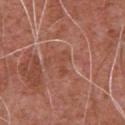| field | value |
|---|---|
| follow-up | no biopsy performed (imaged during a skin exam) |
| body site | the chest |
| lesion diameter | about 3 mm |
| patient | male, aged 73–77 |
| acquisition | 15 mm crop, total-body photography |